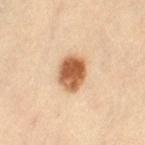Clinical impression:
Captured during whole-body skin photography for melanoma surveillance; the lesion was not biopsied.
Context:
Cropped from a total-body skin-imaging series; the visible field is about 15 mm. A female patient aged around 40. The lesion is on the left thigh. This is a cross-polarized tile.